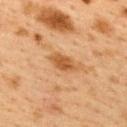follow-up: catalogued during a skin exam; not biopsied | tile lighting: cross-polarized illumination | subject: female, in their 40s | image-analysis metrics: an area of roughly 5.5 mm², an eccentricity of roughly 0.85, and a shape-asymmetry score of about 0.2 (0 = symmetric); a border-irregularity index near 2.5/10 and a within-lesion color-variation index near 2.5/10 | location: the upper back | lesion size: ≈4 mm | imaging modality: ~15 mm crop, total-body skin-cancer survey.